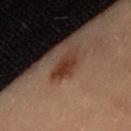  biopsy_status: not biopsied; imaged during a skin examination
  site: lower back
  image:
    source: total-body photography crop
    field_of_view_mm: 15
  automated_metrics:
    area_mm2_approx: 6.0
    eccentricity: 0.9
    shape_asymmetry: 0.3
    border_irregularity_0_10: 3.5
    color_variation_0_10: 4.0
    peripheral_color_asymmetry: 1.0
  lesion_size:
    long_diameter_mm_approx: 4.0
  patient:
    sex: female
    age_approx: 35
  lighting: cross-polarized